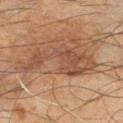biopsy status: catalogued during a skin exam; not biopsied
site: the left thigh
patient: male, approximately 65 years of age
acquisition: total-body-photography crop, ~15 mm field of view